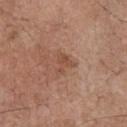| field | value |
|---|---|
| image | ~15 mm tile from a whole-body skin photo |
| subject | male, in their 70s |
| location | the front of the torso |